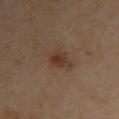The lesion was photographed on a routine skin check and not biopsied; there is no pathology result.
A 15 mm close-up tile from a total-body photography series done for melanoma screening.
This is a cross-polarized tile.
Longest diameter approximately 4.5 mm.
The lesion is located on the chest.
The subject is a female in their 40s.
An algorithmic analysis of the crop reported a lesion area of about 7 mm² and a symmetry-axis asymmetry near 0.3. The software also gave border irregularity of about 3.5 on a 0–10 scale, internal color variation of about 5 on a 0–10 scale, and peripheral color asymmetry of about 1.5. It also reported an automated nevus-likeness rating near 60 out of 100.